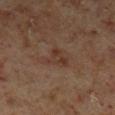This lesion was catalogued during total-body skin photography and was not selected for biopsy. A male subject about 60 years old. Imaged with cross-polarized lighting. On the left lower leg. A close-up tile cropped from a whole-body skin photograph, about 15 mm across. About 3.5 mm across. An algorithmic analysis of the crop reported a footprint of about 6 mm² and an eccentricity of roughly 0.55. The analysis additionally found a border-irregularity index near 5/10 and internal color variation of about 4 on a 0–10 scale.